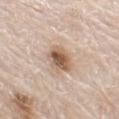Clinical impression:
Captured during whole-body skin photography for melanoma surveillance; the lesion was not biopsied.
Image and clinical context:
From the left thigh. A lesion tile, about 15 mm wide, cut from a 3D total-body photograph. The patient is a male aged 78 to 82. The recorded lesion diameter is about 4 mm.